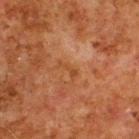notes: total-body-photography surveillance lesion; no biopsy | subject: male, aged 78 to 82 | imaging modality: ~15 mm tile from a whole-body skin photo | location: the upper back.